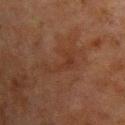No biopsy was performed on this lesion — it was imaged during a full skin examination and was not determined to be concerning.
From the upper back.
Measured at roughly 5.5 mm in maximum diameter.
Cropped from a whole-body photographic skin survey; the tile spans about 15 mm.
An algorithmic analysis of the crop reported a lesion area of about 7.5 mm² and an outline eccentricity of about 0.9 (0 = round, 1 = elongated). It also reported a lesion–skin lightness drop of about 4 and a normalized border contrast of about 5. The analysis additionally found border irregularity of about 9 on a 0–10 scale, a color-variation rating of about 1.5/10, and a peripheral color-asymmetry measure near 0.5. The analysis additionally found a detector confidence of about 100 out of 100 that the crop contains a lesion.
Imaged with cross-polarized lighting.
The patient is a male approximately 60 years of age.